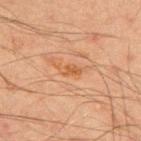{"biopsy_status": "not biopsied; imaged during a skin examination"}